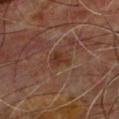biopsy status = catalogued during a skin exam; not biopsied | imaging modality = ~15 mm tile from a whole-body skin photo | image-analysis metrics = a border-irregularity index near 3.5/10, internal color variation of about 1.5 on a 0–10 scale, and peripheral color asymmetry of about 0.5; a nevus-likeness score of about 5/100 and lesion-presence confidence of about 100/100 | anatomic site = the front of the torso | subject = male, in their mid-60s | lighting = cross-polarized.